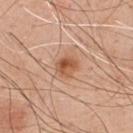Clinical impression:
Part of a total-body skin-imaging series; this lesion was reviewed on a skin check and was not flagged for biopsy.
Context:
A lesion tile, about 15 mm wide, cut from a 3D total-body photograph. The recorded lesion diameter is about 2.5 mm. Automated image analysis of the tile measured a nevus-likeness score of about 90/100. The lesion is on the front of the torso. A male subject aged approximately 50. This is a white-light tile.